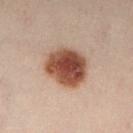The lesion was tiled from a total-body skin photograph and was not biopsied. The patient is a male roughly 50 years of age. Located on the left lower leg. A 15 mm close-up extracted from a 3D total-body photography capture.